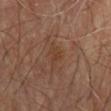Q: Is there a histopathology result?
A: imaged on a skin check; not biopsied
Q: What did automated image analysis measure?
A: a symmetry-axis asymmetry near 0.4; a mean CIELAB color near L≈38 a*≈18 b*≈29 and about 5 CIELAB-L* units darker than the surrounding skin; a border-irregularity rating of about 4.5/10 and a peripheral color-asymmetry measure near 0; a nevus-likeness score of about 0/100 and a detector confidence of about 100 out of 100 that the crop contains a lesion
Q: What are the patient's age and sex?
A: male, aged 68 to 72
Q: What is the imaging modality?
A: total-body-photography crop, ~15 mm field of view
Q: Lesion size?
A: about 3 mm
Q: What is the anatomic site?
A: the mid back